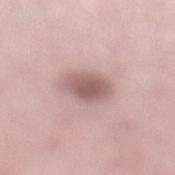Assessment: The lesion was photographed on a routine skin check and not biopsied; there is no pathology result. Image and clinical context: An algorithmic analysis of the crop reported a border-irregularity rating of about 2/10 and a within-lesion color-variation index near 3/10. The recorded lesion diameter is about 4 mm. On the leg. A close-up tile cropped from a whole-body skin photograph, about 15 mm across. The patient is a female roughly 25 years of age. The tile uses white-light illumination.